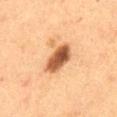biopsy_status: not biopsied; imaged during a skin examination